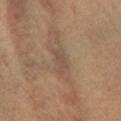The lesion was photographed on a routine skin check and not biopsied; there is no pathology result.
The lesion is located on the leg.
A 15 mm close-up extracted from a 3D total-body photography capture.
A female patient, approximately 30 years of age.